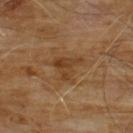Findings:
• biopsy status: catalogued during a skin exam; not biopsied
• illumination: cross-polarized illumination
• diameter: ~3.5 mm (longest diameter)
• location: the chest
• TBP lesion metrics: an outline eccentricity of about 0.35 (0 = round, 1 = elongated); an average lesion color of about L≈38 a*≈18 b*≈33 (CIELAB) and about 7 CIELAB-L* units darker than the surrounding skin; a border-irregularity index near 5.5/10 and internal color variation of about 4 on a 0–10 scale; a classifier nevus-likeness of about 0/100 and a detector confidence of about 100 out of 100 that the crop contains a lesion
• patient: male, aged around 60
• acquisition: 15 mm crop, total-body photography